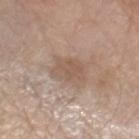notes — imaged on a skin check; not biopsied | imaging modality — total-body-photography crop, ~15 mm field of view | size — ~4 mm (longest diameter) | anatomic site — the left forearm | patient — male, roughly 80 years of age.